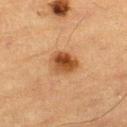Q: Was a biopsy performed?
A: total-body-photography surveillance lesion; no biopsy
Q: Lesion size?
A: ≈3.5 mm
Q: How was the tile lit?
A: cross-polarized
Q: How was this image acquired?
A: total-body-photography crop, ~15 mm field of view
Q: Automated lesion metrics?
A: a footprint of about 8 mm², a shape eccentricity near 0.6, and two-axis asymmetry of about 0.15; a nevus-likeness score of about 100/100
Q: What are the patient's age and sex?
A: male, aged approximately 85 or older
Q: What is the anatomic site?
A: the left thigh